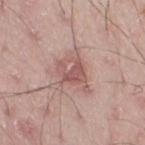<tbp_lesion>
  <biopsy_status>not biopsied; imaged during a skin examination</biopsy_status>
  <lighting>white-light</lighting>
  <site>right thigh</site>
  <image>
    <source>total-body photography crop</source>
    <field_of_view_mm>15</field_of_view_mm>
  </image>
  <patient>
    <sex>male</sex>
    <age_approx>50</age_approx>
  </patient>
</tbp_lesion>